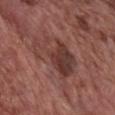No biopsy was performed on this lesion — it was imaged during a full skin examination and was not determined to be concerning. Captured under white-light illumination. From the front of the torso. The subject is a male aged 73 to 77. This image is a 15 mm lesion crop taken from a total-body photograph.